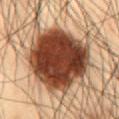Findings:
– workup: total-body-photography surveillance lesion; no biopsy
– lighting: cross-polarized illumination
– patient: male, about 55 years old
– size: about 9.5 mm
– acquisition: 15 mm crop, total-body photography
– anatomic site: the abdomen